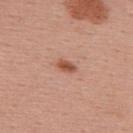Q: Was this lesion biopsied?
A: no biopsy performed (imaged during a skin exam)
Q: Lesion location?
A: the upper back
Q: How was this image acquired?
A: ~15 mm tile from a whole-body skin photo
Q: Patient demographics?
A: female, aged around 55
Q: How was the tile lit?
A: white-light